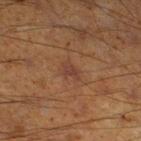Impression:
Part of a total-body skin-imaging series; this lesion was reviewed on a skin check and was not flagged for biopsy.
Background:
An algorithmic analysis of the crop reported an area of roughly 3 mm², a shape eccentricity near 0.8, and two-axis asymmetry of about 0.4. And it measured about 5 CIELAB-L* units darker than the surrounding skin and a normalized lesion–skin contrast near 6. And it measured a border-irregularity rating of about 4/10, a within-lesion color-variation index near 1/10, and peripheral color asymmetry of about 0.5. A male patient, about 60 years old. A region of skin cropped from a whole-body photographic capture, roughly 15 mm wide. Measured at roughly 2.5 mm in maximum diameter. Located on the right thigh. The tile uses cross-polarized illumination.